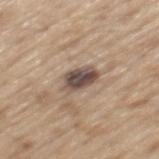{"biopsy_status": "not biopsied; imaged during a skin examination", "lighting": "white-light", "image": {"source": "total-body photography crop", "field_of_view_mm": 15}, "site": "mid back", "patient": {"sex": "male", "age_approx": 70}, "lesion_size": {"long_diameter_mm_approx": 4.0}}